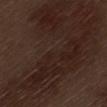Assessment: This lesion was catalogued during total-body skin photography and was not selected for biopsy. Context: Located on the left thigh. A male subject roughly 70 years of age. About 22 mm across. This is a white-light tile. A 15 mm crop from a total-body photograph taken for skin-cancer surveillance. Automated tile analysis of the lesion measured a shape eccentricity near 0.9. It also reported a lesion–skin lightness drop of about 6 and a normalized border contrast of about 7. And it measured a border-irregularity index near 7/10, a color-variation rating of about 4.5/10, and radial color variation of about 1.5. The analysis additionally found a lesion-detection confidence of about 100/100.